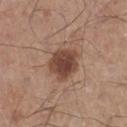Q: Is there a histopathology result?
A: no biopsy performed (imaged during a skin exam)
Q: Lesion location?
A: the left lower leg
Q: Lesion size?
A: about 4 mm
Q: Patient demographics?
A: male, about 60 years old
Q: Illumination type?
A: white-light illumination
Q: What kind of image is this?
A: ~15 mm tile from a whole-body skin photo